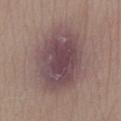Clinical summary: About 9 mm across. On the right lower leg. A region of skin cropped from a whole-body photographic capture, roughly 15 mm wide. A male patient, in their 30s.